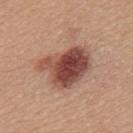Assessment:
Part of a total-body skin-imaging series; this lesion was reviewed on a skin check and was not flagged for biopsy.
Clinical summary:
Imaged with white-light lighting. The patient is a female in their mid- to late 50s. A 15 mm crop from a total-body photograph taken for skin-cancer surveillance. Located on the upper back.